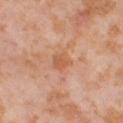The lesion was photographed on a routine skin check and not biopsied; there is no pathology result. An algorithmic analysis of the crop reported a lesion area of about 4.5 mm², a shape eccentricity near 0.65, and two-axis asymmetry of about 0.25. And it measured a lesion color around L≈59 a*≈24 b*≈35 in CIELAB, roughly 7 lightness units darker than nearby skin, and a lesion-to-skin contrast of about 5.5 (normalized; higher = more distinct). And it measured a border-irregularity index near 2.5/10, a color-variation rating of about 2.5/10, and a peripheral color-asymmetry measure near 1. And it measured an automated nevus-likeness rating near 0 out of 100 and a lesion-detection confidence of about 100/100. The subject is a female aged 53–57. From the right thigh. Measured at roughly 3 mm in maximum diameter. A region of skin cropped from a whole-body photographic capture, roughly 15 mm wide.